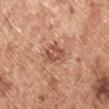No biopsy was performed on this lesion — it was imaged during a full skin examination and was not determined to be concerning. The lesion-visualizer software estimated a nevus-likeness score of about 0/100 and a lesion-detection confidence of about 100/100. A male patient in their mid- to late 50s. This image is a 15 mm lesion crop taken from a total-body photograph. From the arm. About 3.5 mm across. The tile uses white-light illumination.